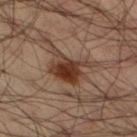Impression: The lesion was photographed on a routine skin check and not biopsied; there is no pathology result. Clinical summary: The patient is a male in their mid- to late 30s. The lesion is located on the left lower leg. This image is a 15 mm lesion crop taken from a total-body photograph.